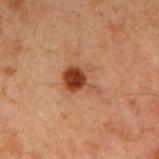Part of a total-body skin-imaging series; this lesion was reviewed on a skin check and was not flagged for biopsy. This is a cross-polarized tile. A 15 mm close-up extracted from a 3D total-body photography capture. The lesion's longest dimension is about 4 mm. The subject is a male aged 58–62. On the right upper arm. An algorithmic analysis of the crop reported a classifier nevus-likeness of about 95/100.